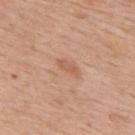| field | value |
|---|---|
| imaging modality | ~15 mm crop, total-body skin-cancer survey |
| patient | male, aged around 60 |
| automated lesion analysis | a mean CIELAB color near L≈58 a*≈23 b*≈31, about 8 CIELAB-L* units darker than the surrounding skin, and a normalized border contrast of about 5.5; a color-variation rating of about 0/10 and radial color variation of about 0; lesion-presence confidence of about 100/100 |
| site | the back |
| lesion diameter | ≈2.5 mm |